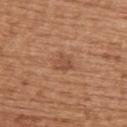workup = total-body-photography surveillance lesion; no biopsy
patient = female, in their mid- to late 60s
diameter = ~2.5 mm (longest diameter)
site = the upper back
tile lighting = white-light
image source = ~15 mm tile from a whole-body skin photo